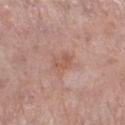The lesion was photographed on a routine skin check and not biopsied; there is no pathology result. Located on the left lower leg. The recorded lesion diameter is about 3 mm. A lesion tile, about 15 mm wide, cut from a 3D total-body photograph. A female patient aged approximately 50. Imaged with white-light lighting.